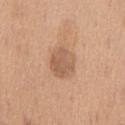Q: How was the tile lit?
A: white-light illumination
Q: Where on the body is the lesion?
A: the chest
Q: How was this image acquired?
A: 15 mm crop, total-body photography
Q: What did automated image analysis measure?
A: an area of roughly 10 mm², a shape eccentricity near 0.65, and a symmetry-axis asymmetry near 0.15; a lesion color around L≈58 a*≈19 b*≈32 in CIELAB, roughly 10 lightness units darker than nearby skin, and a normalized border contrast of about 6.5; a lesion-detection confidence of about 100/100
Q: What is the lesion's diameter?
A: ≈4 mm
Q: What are the patient's age and sex?
A: male, approximately 60 years of age